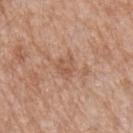Imaged during a routine full-body skin examination; the lesion was not biopsied and no histopathology is available. A male subject, in their 50s. The recorded lesion diameter is about 2.5 mm. The lesion is located on the arm. A 15 mm close-up extracted from a 3D total-body photography capture. The tile uses white-light illumination.